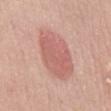Imaged during a routine full-body skin examination; the lesion was not biopsied and no histopathology is available. On the mid back. A 15 mm close-up extracted from a 3D total-body photography capture. A female patient, aged around 65. An algorithmic analysis of the crop reported an area of roughly 21 mm², an outline eccentricity of about 0.75 (0 = round, 1 = elongated), and a shape-asymmetry score of about 0.15 (0 = symmetric).